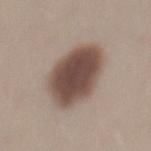patient: male, in their 30s
imaging modality: ~15 mm crop, total-body skin-cancer survey
anatomic site: the mid back
illumination: white-light illumination
diameter: about 7 mm